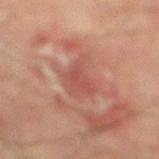On the right lower leg.
A region of skin cropped from a whole-body photographic capture, roughly 15 mm wide.
Captured under cross-polarized illumination.
The subject is a male aged 73–77.
Approximately 4.5 mm at its widest.
The total-body-photography lesion software estimated a footprint of about 10 mm², an eccentricity of roughly 0.7, and a symmetry-axis asymmetry near 0.35. The analysis additionally found border irregularity of about 4.5 on a 0–10 scale and a within-lesion color-variation index near 2.5/10.